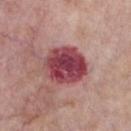Case summary:
* biopsy status · catalogued during a skin exam; not biopsied
* lighting · white-light illumination
* location · the front of the torso
* automated metrics · roughly 17 lightness units darker than nearby skin and a lesion-to-skin contrast of about 12.5 (normalized; higher = more distinct); a color-variation rating of about 7/10 and peripheral color asymmetry of about 2; an automated nevus-likeness rating near 0 out of 100
* lesion diameter · ~5 mm (longest diameter)
* imaging modality · ~15 mm crop, total-body skin-cancer survey
* patient · male, in their mid- to late 70s